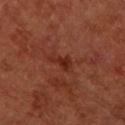Clinical impression: Recorded during total-body skin imaging; not selected for excision or biopsy. Acquisition and patient details: A 15 mm crop from a total-body photograph taken for skin-cancer surveillance. A female subject aged around 65. About 2.5 mm across. On the left forearm.